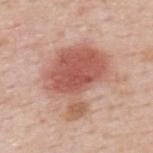Assessment: This lesion was catalogued during total-body skin photography and was not selected for biopsy. Clinical summary: The total-body-photography lesion software estimated a lesion color around L≈57 a*≈25 b*≈28 in CIELAB and a normalized lesion–skin contrast near 8.5. The analysis additionally found an automated nevus-likeness rating near 100 out of 100. A 15 mm close-up extracted from a 3D total-body photography capture. Captured under white-light illumination. The lesion is on the upper back. Measured at roughly 7.5 mm in maximum diameter. A male patient aged around 50.